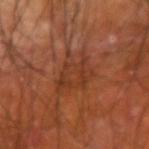  biopsy_status: not biopsied; imaged during a skin examination
  site: right upper arm
  patient:
    sex: male
    age_approx: 60
  image:
    source: total-body photography crop
    field_of_view_mm: 15
  lighting: cross-polarized
  lesion_size:
    long_diameter_mm_approx: 4.5